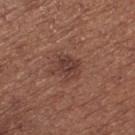lesion diameter: ~3 mm (longest diameter); imaging modality: total-body-photography crop, ~15 mm field of view; patient: female, aged 53 to 57; body site: the left thigh; illumination: white-light illumination.